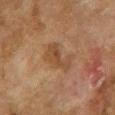The lesion was photographed on a routine skin check and not biopsied; there is no pathology result. An algorithmic analysis of the crop reported a lesion color around L≈41 a*≈18 b*≈31 in CIELAB, a lesion–skin lightness drop of about 7, and a normalized border contrast of about 6.5. It also reported a border-irregularity rating of about 5.5/10, a color-variation rating of about 3/10, and a peripheral color-asymmetry measure near 1. The tile uses cross-polarized illumination. The recorded lesion diameter is about 4 mm. The patient is a female about 60 years old. From the right upper arm. A roughly 15 mm field-of-view crop from a total-body skin photograph.